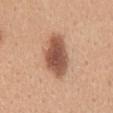The lesion was photographed on a routine skin check and not biopsied; there is no pathology result.
An algorithmic analysis of the crop reported a lesion color around L≈54 a*≈22 b*≈30 in CIELAB and a normalized border contrast of about 10.5. And it measured a nevus-likeness score of about 95/100 and a detector confidence of about 100 out of 100 that the crop contains a lesion.
A female subject, aged approximately 30.
A region of skin cropped from a whole-body photographic capture, roughly 15 mm wide.
The lesion is on the mid back.